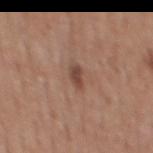Recorded during total-body skin imaging; not selected for excision or biopsy. From the mid back. A male patient, approximately 55 years of age. Approximately 2.5 mm at its widest. The lesion-visualizer software estimated a lesion area of about 3.5 mm² and an eccentricity of roughly 0.85. And it measured an automated nevus-likeness rating near 75 out of 100. Imaged with white-light lighting. A 15 mm crop from a total-body photograph taken for skin-cancer surveillance.